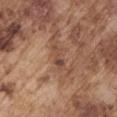<lesion>
<biopsy_status>not biopsied; imaged during a skin examination</biopsy_status>
<image>
  <source>total-body photography crop</source>
  <field_of_view_mm>15</field_of_view_mm>
</image>
<automated_metrics>
  <area_mm2_approx>3.0</area_mm2_approx>
  <eccentricity>0.95</eccentricity>
  <shape_asymmetry>0.45</shape_asymmetry>
  <color_variation_0_10>0.0</color_variation_0_10>
  <peripheral_color_asymmetry>0.0</peripheral_color_asymmetry>
  <nevus_likeness_0_100>0</nevus_likeness_0_100>
</automated_metrics>
<patient>
  <sex>male</sex>
  <age_approx>75</age_approx>
</patient>
<site>right upper arm</site>
</lesion>